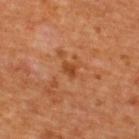Recorded during total-body skin imaging; not selected for excision or biopsy. The tile uses cross-polarized illumination. Measured at roughly 2 mm in maximum diameter. Located on the back. This image is a 15 mm lesion crop taken from a total-body photograph. Automated image analysis of the tile measured a shape-asymmetry score of about 0.35 (0 = symmetric). The analysis additionally found a mean CIELAB color near L≈42 a*≈27 b*≈37 and a lesion–skin lightness drop of about 8. The analysis additionally found lesion-presence confidence of about 100/100. The subject is a female about 55 years old.